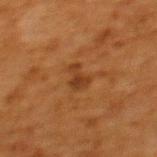Part of a total-body skin-imaging series; this lesion was reviewed on a skin check and was not flagged for biopsy. Approximately 3 mm at its widest. Captured under cross-polarized illumination. A female patient, aged 48–52. A close-up tile cropped from a whole-body skin photograph, about 15 mm across. Automated tile analysis of the lesion measured an average lesion color of about L≈32 a*≈21 b*≈31 (CIELAB) and roughly 7 lightness units darker than nearby skin. The analysis additionally found a border-irregularity index near 5/10. The lesion is on the upper back.